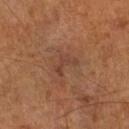follow-up — imaged on a skin check; not biopsied | subject — male, aged 63–67 | automated lesion analysis — a lesion color around L≈40 a*≈21 b*≈28 in CIELAB and a normalized lesion–skin contrast near 5; a nevus-likeness score of about 0/100 | size — about 3 mm | illumination — cross-polarized illumination | imaging modality — ~15 mm tile from a whole-body skin photo.